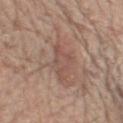The lesion was tiled from a total-body skin photograph and was not biopsied. Located on the chest. The patient is a male approximately 80 years of age. Cropped from a whole-body photographic skin survey; the tile spans about 15 mm.